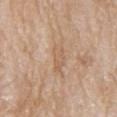<case>
<biopsy_status>not biopsied; imaged during a skin examination</biopsy_status>
<patient>
  <sex>male</sex>
  <age_approx>85+</age_approx>
</patient>
<automated_metrics>
  <area_mm2_approx>5.0</area_mm2_approx>
  <eccentricity>0.9</eccentricity>
  <shape_asymmetry>0.3</shape_asymmetry>
  <cielab_L>60</cielab_L>
  <cielab_a>18</cielab_a>
  <cielab_b>32</cielab_b>
  <vs_skin_darker_L>6.0</vs_skin_darker_L>
</automated_metrics>
<image>
  <source>total-body photography crop</source>
  <field_of_view_mm>15</field_of_view_mm>
</image>
<site>back</site>
</case>